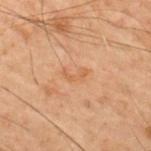No biopsy was performed on this lesion — it was imaged during a full skin examination and was not determined to be concerning.
The lesion is on the upper back.
A lesion tile, about 15 mm wide, cut from a 3D total-body photograph.
A male patient approximately 60 years of age.
The tile uses cross-polarized illumination.
The lesion-visualizer software estimated a mean CIELAB color near L≈46 a*≈18 b*≈30, about 5 CIELAB-L* units darker than the surrounding skin, and a normalized lesion–skin contrast near 4.5. The analysis additionally found a lesion-detection confidence of about 100/100.
Longest diameter approximately 3 mm.